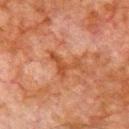Findings:
– notes · imaged on a skin check; not biopsied
– subject · male, approximately 80 years of age
– imaging modality · 15 mm crop, total-body photography
– lesion diameter · about 4 mm
– tile lighting · cross-polarized
– body site · the chest